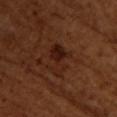notes: no biopsy performed (imaged during a skin exam).